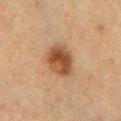Clinical impression:
Captured during whole-body skin photography for melanoma surveillance; the lesion was not biopsied.
Clinical summary:
A female subject, in their mid- to late 50s. A 15 mm close-up tile from a total-body photography series done for melanoma screening. Automated tile analysis of the lesion measured an area of roughly 11 mm², a shape eccentricity near 0.7, and a symmetry-axis asymmetry near 0.15. It also reported a detector confidence of about 100 out of 100 that the crop contains a lesion. Located on the chest.